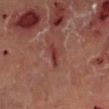Assessment: Recorded during total-body skin imaging; not selected for excision or biopsy. Clinical summary: The lesion is located on the left lower leg. Imaged with cross-polarized lighting. The subject is a male aged approximately 55. A 15 mm close-up extracted from a 3D total-body photography capture.